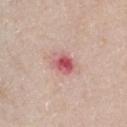The lesion is located on the front of the torso.
The tile uses white-light illumination.
The subject is a male about 40 years old.
This image is a 15 mm lesion crop taken from a total-body photograph.
About 3 mm across.